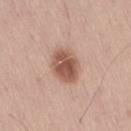follow-up=catalogued during a skin exam; not biopsied | anatomic site=the right thigh | lesion diameter=~4 mm (longest diameter) | patient=male, about 55 years old | imaging modality=15 mm crop, total-body photography | illumination=white-light illumination | image-analysis metrics=an area of roughly 10 mm² and two-axis asymmetry of about 0.1; a mean CIELAB color near L≈54 a*≈22 b*≈28, a lesion–skin lightness drop of about 14, and a normalized lesion–skin contrast near 9.5; a border-irregularity rating of about 1/10, a color-variation rating of about 4.5/10, and a peripheral color-asymmetry measure near 1.5.